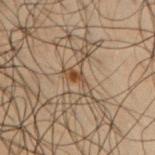| field | value |
|---|---|
| workup | imaged on a skin check; not biopsied |